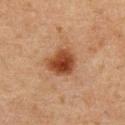Assessment:
This lesion was catalogued during total-body skin photography and was not selected for biopsy.
Background:
Located on the abdomen. A 15 mm crop from a total-body photograph taken for skin-cancer surveillance. An algorithmic analysis of the crop reported a nevus-likeness score of about 100/100 and lesion-presence confidence of about 100/100. This is a cross-polarized tile. The patient is a male in their mid-70s.